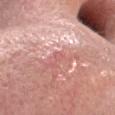* biopsy status · no biopsy performed (imaged during a skin exam)
* anatomic site · the head or neck
* imaging modality · total-body-photography crop, ~15 mm field of view
* tile lighting · white-light
* lesion size · about 1 mm
* subject · female, aged around 70
* image-analysis metrics · a symmetry-axis asymmetry near 0.3; a lesion–skin lightness drop of about 6; a classifier nevus-likeness of about 0/100 and lesion-presence confidence of about 85/100